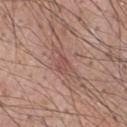Impression:
The lesion was photographed on a routine skin check and not biopsied; there is no pathology result.